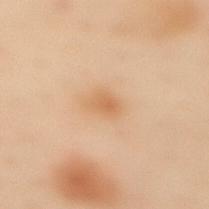| field | value |
|---|---|
| notes | catalogued during a skin exam; not biopsied |
| TBP lesion metrics | roughly 7 lightness units darker than nearby skin and a normalized border contrast of about 6; a nevus-likeness score of about 40/100 |
| tile lighting | cross-polarized illumination |
| imaging modality | ~15 mm tile from a whole-body skin photo |
| patient | female, aged approximately 60 |
| location | the mid back |